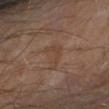Case summary:
– biopsy status · imaged on a skin check; not biopsied
– image source · ~15 mm tile from a whole-body skin photo
– lighting · cross-polarized
– site · the right forearm
– subject · male, roughly 65 years of age